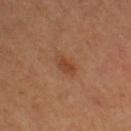| key | value |
|---|---|
| workup | imaged on a skin check; not biopsied |
| subject | female, aged around 60 |
| image-analysis metrics | a lesion area of about 4 mm² and a symmetry-axis asymmetry near 0.2; a mean CIELAB color near L≈45 a*≈24 b*≈35 and roughly 8 lightness units darker than nearby skin; a nevus-likeness score of about 65/100 and lesion-presence confidence of about 100/100 |
| imaging modality | ~15 mm tile from a whole-body skin photo |
| body site | the right thigh |
| size | about 3 mm |
| illumination | cross-polarized |